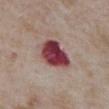Part of a total-body skin-imaging series; this lesion was reviewed on a skin check and was not flagged for biopsy. A male patient, in their mid-70s. The lesion is on the abdomen. A region of skin cropped from a whole-body photographic capture, roughly 15 mm wide.